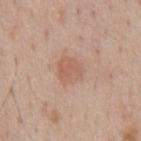Acquisition and patient details: A 15 mm crop from a total-body photograph taken for skin-cancer surveillance. Located on the chest. A male patient, aged 58–62. The tile uses white-light illumination.